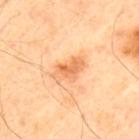Part of a total-body skin-imaging series; this lesion was reviewed on a skin check and was not flagged for biopsy. A 15 mm close-up tile from a total-body photography series done for melanoma screening. A male patient, about 40 years old. The lesion is on the upper back.